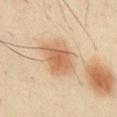Part of a total-body skin-imaging series; this lesion was reviewed on a skin check and was not flagged for biopsy. Captured under cross-polarized illumination. A 15 mm crop from a total-body photograph taken for skin-cancer surveillance. Located on the chest. A male patient, about 50 years old.